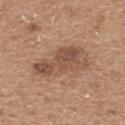Assessment: This lesion was catalogued during total-body skin photography and was not selected for biopsy. Background: A 15 mm close-up extracted from a 3D total-body photography capture. Imaged with white-light lighting. About 7 mm across. The lesion is on the upper back. The subject is a male aged 63–67.